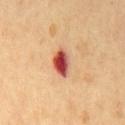{
  "biopsy_status": "not biopsied; imaged during a skin examination",
  "site": "chest",
  "automated_metrics": {
    "cielab_L": 54,
    "cielab_a": 33,
    "cielab_b": 33,
    "vs_skin_darker_L": 19.0,
    "vs_skin_contrast_norm": 12.5,
    "border_irregularity_0_10": 2.0,
    "nevus_likeness_0_100": 0,
    "lesion_detection_confidence_0_100": 100
  },
  "image": {
    "source": "total-body photography crop",
    "field_of_view_mm": 15
  },
  "patient": {
    "sex": "male",
    "age_approx": 50
  },
  "lighting": "cross-polarized"
}